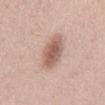Part of a total-body skin-imaging series; this lesion was reviewed on a skin check and was not flagged for biopsy. The lesion is located on the mid back. A female patient aged approximately 60. A 15 mm crop from a total-body photograph taken for skin-cancer surveillance.